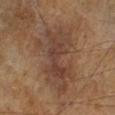{
  "biopsy_status": "not biopsied; imaged during a skin examination",
  "patient": {
    "sex": "male",
    "age_approx": 65
  },
  "image": {
    "source": "total-body photography crop",
    "field_of_view_mm": 15
  },
  "automated_metrics": {
    "area_mm2_approx": 28.0,
    "eccentricity": 0.85,
    "shape_asymmetry": 0.4,
    "vs_skin_contrast_norm": 7.0
  },
  "lesion_size": {
    "long_diameter_mm_approx": 8.5
  },
  "site": "left lower leg"
}